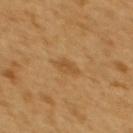Part of a total-body skin-imaging series; this lesion was reviewed on a skin check and was not flagged for biopsy. Captured under cross-polarized illumination. The total-body-photography lesion software estimated a footprint of about 3.5 mm², an outline eccentricity of about 0.8 (0 = round, 1 = elongated), and two-axis asymmetry of about 0.25. The analysis additionally found a mean CIELAB color near L≈51 a*≈19 b*≈41, a lesion–skin lightness drop of about 7, and a lesion-to-skin contrast of about 5.5 (normalized; higher = more distinct). The software also gave an automated nevus-likeness rating near 5 out of 100 and a detector confidence of about 100 out of 100 that the crop contains a lesion. From the upper back. The patient is a female approximately 55 years of age. A roughly 15 mm field-of-view crop from a total-body skin photograph.